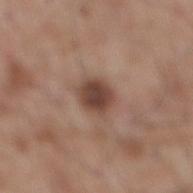The lesion was photographed on a routine skin check and not biopsied; there is no pathology result.
Automated image analysis of the tile measured a footprint of about 7 mm² and a shape-asymmetry score of about 0.1 (0 = symmetric). The analysis additionally found internal color variation of about 3.5 on a 0–10 scale and a peripheral color-asymmetry measure near 1. It also reported a lesion-detection confidence of about 100/100.
Imaged with white-light lighting.
A close-up tile cropped from a whole-body skin photograph, about 15 mm across.
The lesion is on the mid back.
A male subject, aged around 60.
About 3 mm across.